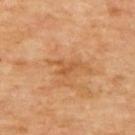Assessment:
No biopsy was performed on this lesion — it was imaged during a full skin examination and was not determined to be concerning.
Acquisition and patient details:
Longest diameter approximately 4 mm. A 15 mm close-up tile from a total-body photography series done for melanoma screening. The patient is in their mid-60s. Imaged with cross-polarized lighting. The lesion is on the upper back.